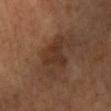Assessment:
The lesion was tiled from a total-body skin photograph and was not biopsied.
Context:
The lesion is on the left lower leg. A female subject about 65 years old. Automated tile analysis of the lesion measured an outline eccentricity of about 0.55 (0 = round, 1 = elongated) and a shape-asymmetry score of about 0.4 (0 = symmetric). It also reported an average lesion color of about L≈31 a*≈18 b*≈26 (CIELAB), roughly 7 lightness units darker than nearby skin, and a normalized border contrast of about 7. The analysis additionally found an automated nevus-likeness rating near 0 out of 100 and a lesion-detection confidence of about 100/100. A roughly 15 mm field-of-view crop from a total-body skin photograph. Approximately 3.5 mm at its widest. Captured under cross-polarized illumination.